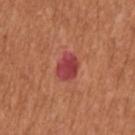notes = no biopsy performed (imaged during a skin exam)
automated metrics = an eccentricity of roughly 0.65 and two-axis asymmetry of about 0.2; an average lesion color of about L≈44 a*≈36 b*≈25 (CIELAB), about 11 CIELAB-L* units darker than the surrounding skin, and a normalized border contrast of about 10
anatomic site = the arm
diameter = about 3 mm
image = total-body-photography crop, ~15 mm field of view
illumination = white-light illumination
subject = female, aged approximately 65